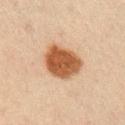The lesion was photographed on a routine skin check and not biopsied; there is no pathology result. The lesion is located on the right upper arm. A male subject about 35 years old. A lesion tile, about 15 mm wide, cut from a 3D total-body photograph.